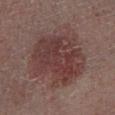Captured during whole-body skin photography for melanoma surveillance; the lesion was not biopsied.
The subject is a male aged approximately 50.
On the left lower leg.
Approximately 8.5 mm at its widest.
A 15 mm close-up extracted from a 3D total-body photography capture.